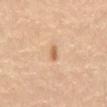Assessment:
The lesion was photographed on a routine skin check and not biopsied; there is no pathology result.
Acquisition and patient details:
The subject is a male aged 53 to 57. A close-up tile cropped from a whole-body skin photograph, about 15 mm across. The total-body-photography lesion software estimated a footprint of about 2 mm² and a shape eccentricity near 0.8. The software also gave a color-variation rating of about 0/10 and peripheral color asymmetry of about 0. The analysis additionally found an automated nevus-likeness rating near 75 out of 100 and a detector confidence of about 100 out of 100 that the crop contains a lesion. Located on the abdomen.